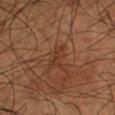Recorded during total-body skin imaging; not selected for excision or biopsy. Located on the left forearm. A roughly 15 mm field-of-view crop from a total-body skin photograph. A male subject approximately 55 years of age.